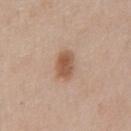follow-up = catalogued during a skin exam; not biopsied | patient = male, aged around 60 | anatomic site = the mid back | imaging modality = 15 mm crop, total-body photography.